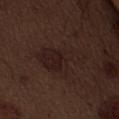Impression: Imaged during a routine full-body skin examination; the lesion was not biopsied and no histopathology is available. Acquisition and patient details: This image is a 15 mm lesion crop taken from a total-body photograph. A male subject roughly 70 years of age. The lesion is located on the abdomen.